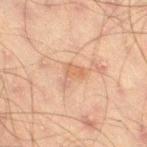image:
  source: total-body photography crop
  field_of_view_mm: 15
automated_metrics:
  cielab_L: 53
  cielab_a: 18
  cielab_b: 29
  vs_skin_darker_L: 7.0
  nevus_likeness_0_100: 0
patient:
  sex: male
  age_approx: 45
site: right thigh